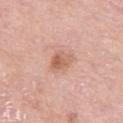biopsy status: total-body-photography surveillance lesion; no biopsy | lighting: white-light | body site: the right thigh | patient: female, roughly 70 years of age | image source: ~15 mm crop, total-body skin-cancer survey | size: about 3 mm | TBP lesion metrics: an area of roughly 6 mm², an outline eccentricity of about 0.65 (0 = round, 1 = elongated), and a symmetry-axis asymmetry near 0.25; a lesion color around L≈62 a*≈23 b*≈30 in CIELAB, a lesion–skin lightness drop of about 9, and a lesion-to-skin contrast of about 6.5 (normalized; higher = more distinct); border irregularity of about 2.5 on a 0–10 scale, a within-lesion color-variation index near 5.5/10, and radial color variation of about 2.